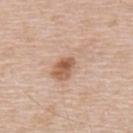{
  "biopsy_status": "not biopsied; imaged during a skin examination",
  "patient": {
    "sex": "male",
    "age_approx": 50
  },
  "image": {
    "source": "total-body photography crop",
    "field_of_view_mm": 15
  },
  "site": "upper back"
}